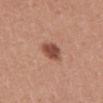Findings:
* biopsy status — total-body-photography surveillance lesion; no biopsy
* image-analysis metrics — a shape eccentricity near 0.45 and two-axis asymmetry of about 0.2; a lesion color around L≈49 a*≈24 b*≈29 in CIELAB and about 14 CIELAB-L* units darker than the surrounding skin
* subject — male, aged around 45
* lighting — white-light
* image source — total-body-photography crop, ~15 mm field of view
* site — the mid back